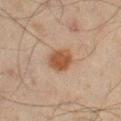biopsy_status: not biopsied; imaged during a skin examination
automated_metrics:
  area_mm2_approx: 7.5
  eccentricity: 0.35
  shape_asymmetry: 0.15
  border_irregularity_0_10: 1.0
  color_variation_0_10: 3.0
site: right thigh
lesion_size:
  long_diameter_mm_approx: 3.0
patient:
  sex: male
  age_approx: 45
image:
  source: total-body photography crop
  field_of_view_mm: 15